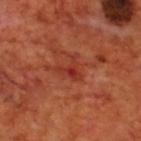Impression:
Part of a total-body skin-imaging series; this lesion was reviewed on a skin check and was not flagged for biopsy.
Acquisition and patient details:
The patient is a male about 70 years old. Approximately 3 mm at its widest. Cropped from a whole-body photographic skin survey; the tile spans about 15 mm. This is a cross-polarized tile. The lesion is located on the upper back.